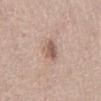Part of a total-body skin-imaging series; this lesion was reviewed on a skin check and was not flagged for biopsy.
Located on the right lower leg.
A female subject in their mid-60s.
The tile uses white-light illumination.
The lesion-visualizer software estimated an area of roughly 4.5 mm² and an eccentricity of roughly 0.75. And it measured roughly 11 lightness units darker than nearby skin and a normalized lesion–skin contrast near 7.5. The analysis additionally found a border-irregularity rating of about 3/10, internal color variation of about 4 on a 0–10 scale, and radial color variation of about 1. The analysis additionally found a classifier nevus-likeness of about 35/100 and a lesion-detection confidence of about 100/100.
Measured at roughly 3 mm in maximum diameter.
A 15 mm close-up tile from a total-body photography series done for melanoma screening.